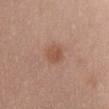The lesion was tiled from a total-body skin photograph and was not biopsied. From the chest. Measured at roughly 2.5 mm in maximum diameter. Captured under white-light illumination. A close-up tile cropped from a whole-body skin photograph, about 15 mm across. The patient is a female aged 48–52.